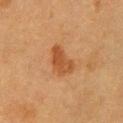Impression: Captured during whole-body skin photography for melanoma surveillance; the lesion was not biopsied. Image and clinical context: This image is a 15 mm lesion crop taken from a total-body photograph. A female patient approximately 55 years of age. On the left upper arm.